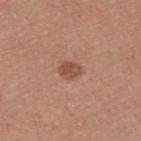The lesion was photographed on a routine skin check and not biopsied; there is no pathology result.
A 15 mm crop from a total-body photograph taken for skin-cancer surveillance.
Approximately 2.5 mm at its widest.
A male patient, aged 38 to 42.
The lesion is located on the left upper arm.